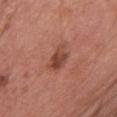Part of a total-body skin-imaging series; this lesion was reviewed on a skin check and was not flagged for biopsy. The lesion is located on the chest. A 15 mm crop from a total-body photograph taken for skin-cancer surveillance. The recorded lesion diameter is about 3.5 mm. A female subject, about 60 years old.